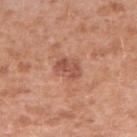| field | value |
|---|---|
| notes | catalogued during a skin exam; not biopsied |
| automated metrics | a footprint of about 6 mm² and two-axis asymmetry of about 0.25; an average lesion color of about L≈53 a*≈26 b*≈30 (CIELAB), roughly 9 lightness units darker than nearby skin, and a normalized border contrast of about 6.5; a border-irregularity rating of about 2.5/10 and a peripheral color-asymmetry measure near 1; a classifier nevus-likeness of about 0/100 and a detector confidence of about 100 out of 100 that the crop contains a lesion |
| subject | female, about 40 years old |
| tile lighting | white-light illumination |
| site | the right upper arm |
| acquisition | ~15 mm crop, total-body skin-cancer survey |
| diameter | ≈3 mm |